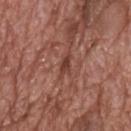<record>
  <biopsy_status>not biopsied; imaged during a skin examination</biopsy_status>
</record>